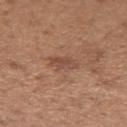Part of a total-body skin-imaging series; this lesion was reviewed on a skin check and was not flagged for biopsy. The lesion is located on the left forearm. A roughly 15 mm field-of-view crop from a total-body skin photograph. The subject is a female aged 28 to 32.